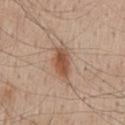{
  "biopsy_status": "not biopsied; imaged during a skin examination",
  "patient": {
    "sex": "male",
    "age_approx": 55
  },
  "lighting": "white-light",
  "site": "mid back",
  "image": {
    "source": "total-body photography crop",
    "field_of_view_mm": 15
  },
  "lesion_size": {
    "long_diameter_mm_approx": 4.5
  },
  "automated_metrics": {
    "area_mm2_approx": 8.5,
    "eccentricity": 0.85,
    "vs_skin_darker_L": 11.0,
    "lesion_detection_confidence_0_100": 100
  }
}